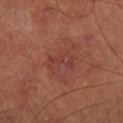The lesion was tiled from a total-body skin photograph and was not biopsied.
The tile uses cross-polarized illumination.
The lesion is on the right lower leg.
An algorithmic analysis of the crop reported a symmetry-axis asymmetry near 0.4. The software also gave a lesion color around L≈39 a*≈27 b*≈25 in CIELAB and roughly 5 lightness units darker than nearby skin. The analysis additionally found an automated nevus-likeness rating near 5 out of 100 and a lesion-detection confidence of about 100/100.
A male subject, about 65 years old.
This image is a 15 mm lesion crop taken from a total-body photograph.